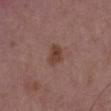Recorded during total-body skin imaging; not selected for excision or biopsy. A male subject roughly 50 years of age. The total-body-photography lesion software estimated an area of roughly 5 mm², an outline eccentricity of about 0.75 (0 = round, 1 = elongated), and a symmetry-axis asymmetry near 0.35. And it measured a normalized lesion–skin contrast near 7.5. It also reported a border-irregularity index near 3/10, a within-lesion color-variation index near 2.5/10, and radial color variation of about 1. The analysis additionally found an automated nevus-likeness rating near 70 out of 100 and a lesion-detection confidence of about 100/100. Located on the chest. A region of skin cropped from a whole-body photographic capture, roughly 15 mm wide. The recorded lesion diameter is about 3 mm. Imaged with white-light lighting.